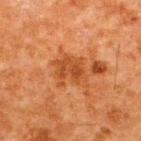Clinical impression:
The lesion was tiled from a total-body skin photograph and was not biopsied.
Clinical summary:
Captured under cross-polarized illumination. A close-up tile cropped from a whole-body skin photograph, about 15 mm across. A male patient, approximately 60 years of age. Located on the upper back.